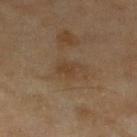Findings:
* notes — catalogued during a skin exam; not biopsied
* patient — female, approximately 60 years of age
* illumination — cross-polarized
* acquisition — ~15 mm crop, total-body skin-cancer survey
* site — the left lower leg